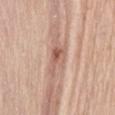Notes:
- biopsy status · total-body-photography surveillance lesion; no biopsy
- lighting · white-light
- body site · the abdomen
- image source · ~15 mm crop, total-body skin-cancer survey
- patient · female, about 65 years old
- size · ≈3.5 mm